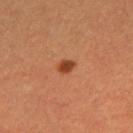Findings:
– biopsy status: catalogued during a skin exam; not biopsied
– image source: total-body-photography crop, ~15 mm field of view
– lesion diameter: ~2 mm (longest diameter)
– subject: female, in their 30s
– site: the left thigh
– TBP lesion metrics: a shape-asymmetry score of about 0.25 (0 = symmetric); border irregularity of about 2 on a 0–10 scale and peripheral color asymmetry of about 0.5